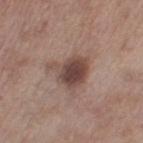image-analysis metrics = a lesion area of about 11 mm², a shape eccentricity near 0.45, and two-axis asymmetry of about 0.35; an average lesion color of about L≈45 a*≈18 b*≈22 (CIELAB), a lesion–skin lightness drop of about 13, and a normalized lesion–skin contrast near 9.5; a nevus-likeness score of about 35/100 and lesion-presence confidence of about 100/100 | site = the left thigh | patient = female, aged 53 to 57 | image source = total-body-photography crop, ~15 mm field of view | tile lighting = white-light.